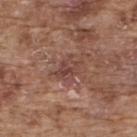  biopsy_status: not biopsied; imaged during a skin examination
  lesion_size:
    long_diameter_mm_approx: 3.5
  site: upper back
  patient:
    sex: male
    age_approx: 75
  image:
    source: total-body photography crop
    field_of_view_mm: 15
  lighting: white-light